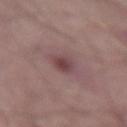Findings:
• biopsy status · no biopsy performed (imaged during a skin exam)
• illumination · white-light
• imaging modality · ~15 mm tile from a whole-body skin photo
• location · the lower back
• subject · male, approximately 65 years of age